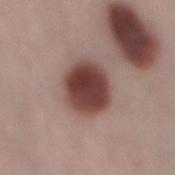This lesion was catalogued during total-body skin photography and was not selected for biopsy. A female patient in their mid- to late 20s. Captured under white-light illumination. From the leg. A lesion tile, about 15 mm wide, cut from a 3D total-body photograph. Approximately 5 mm at its widest.